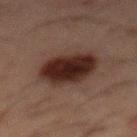<record>
<biopsy_status>not biopsied; imaged during a skin examination</biopsy_status>
<lesion_size>
  <long_diameter_mm_approx>5.5</long_diameter_mm_approx>
</lesion_size>
<patient>
  <sex>male</sex>
  <age_approx>50</age_approx>
</patient>
<lighting>cross-polarized</lighting>
<site>mid back</site>
<automated_metrics>
  <area_mm2_approx>18.0</area_mm2_approx>
  <eccentricity>0.7</eccentricity>
  <shape_asymmetry>0.15</shape_asymmetry>
  <cielab_L>19</cielab_L>
  <cielab_a>15</cielab_a>
  <cielab_b>17</cielab_b>
</automated_metrics>
<image>
  <source>total-body photography crop</source>
  <field_of_view_mm>15</field_of_view_mm>
</image>
</record>